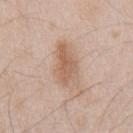  biopsy_status: not biopsied; imaged during a skin examination
  site: chest
  lighting: white-light
  patient:
    sex: male
    age_approx: 45
  lesion_size:
    long_diameter_mm_approx: 5.5
  automated_metrics:
    border_irregularity_0_10: 3.0
    color_variation_0_10: 4.0
    peripheral_color_asymmetry: 1.0
    nevus_likeness_0_100: 40
    lesion_detection_confidence_0_100: 100
  image:
    source: total-body photography crop
    field_of_view_mm: 15